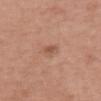Clinical impression: Captured during whole-body skin photography for melanoma surveillance; the lesion was not biopsied. Acquisition and patient details: The lesion's longest dimension is about 2.5 mm. A 15 mm crop from a total-body photograph taken for skin-cancer surveillance. The lesion is located on the upper back. This is a white-light tile. A female patient aged around 70.